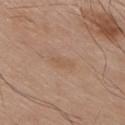The tile uses white-light illumination. Located on the chest. This image is a 15 mm lesion crop taken from a total-body photograph. The recorded lesion diameter is about 2.5 mm. A male subject, roughly 80 years of age.